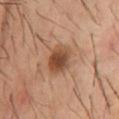Findings:
– notes — imaged on a skin check; not biopsied
– patient — male, aged 58–62
– site — the chest
– acquisition — 15 mm crop, total-body photography
– illumination — cross-polarized